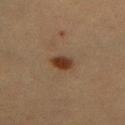A female patient, aged 38 to 42.
Located on the abdomen.
Imaged with cross-polarized lighting.
A close-up tile cropped from a whole-body skin photograph, about 15 mm across.